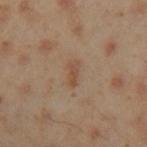follow-up=catalogued during a skin exam; not biopsied
body site=the left upper arm
image source=15 mm crop, total-body photography
size=≈3 mm
patient=male, in their mid- to late 40s